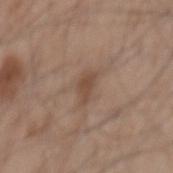Q: Patient demographics?
A: male, about 55 years old
Q: Lesion location?
A: the mid back
Q: What did automated image analysis measure?
A: a lesion color around L≈47 a*≈17 b*≈27 in CIELAB, roughly 8 lightness units darker than nearby skin, and a normalized lesion–skin contrast near 6.5; a border-irregularity index near 4/10, a within-lesion color-variation index near 1/10, and a peripheral color-asymmetry measure near 0.5; a classifier nevus-likeness of about 5/100 and a lesion-detection confidence of about 100/100
Q: What kind of image is this?
A: ~15 mm crop, total-body skin-cancer survey